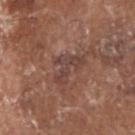Q: Was this lesion biopsied?
A: imaged on a skin check; not biopsied
Q: How large is the lesion?
A: ~4 mm (longest diameter)
Q: What kind of image is this?
A: ~15 mm crop, total-body skin-cancer survey
Q: Where on the body is the lesion?
A: the head or neck
Q: What did automated image analysis measure?
A: a footprint of about 7.5 mm², an eccentricity of roughly 0.75, and a symmetry-axis asymmetry near 0.65; a mean CIELAB color near L≈42 a*≈19 b*≈22, about 7 CIELAB-L* units darker than the surrounding skin, and a lesion-to-skin contrast of about 6.5 (normalized; higher = more distinct); a classifier nevus-likeness of about 0/100 and lesion-presence confidence of about 80/100
Q: What are the patient's age and sex?
A: male, approximately 80 years of age
Q: What lighting was used for the tile?
A: white-light